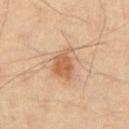Part of a total-body skin-imaging series; this lesion was reviewed on a skin check and was not flagged for biopsy. A close-up tile cropped from a whole-body skin photograph, about 15 mm across. The lesion is on the front of the torso. Measured at roughly 4 mm in maximum diameter. The lesion-visualizer software estimated a classifier nevus-likeness of about 95/100 and lesion-presence confidence of about 100/100. This is a cross-polarized tile. The patient is a male approximately 55 years of age.